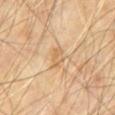notes: imaged on a skin check; not biopsied
site: the chest
subject: male, aged approximately 70
image source: ~15 mm crop, total-body skin-cancer survey